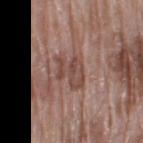Impression: Part of a total-body skin-imaging series; this lesion was reviewed on a skin check and was not flagged for biopsy. Acquisition and patient details: Longest diameter approximately 4 mm. The tile uses white-light illumination. A female patient, about 75 years old. From the right thigh. Automated tile analysis of the lesion measured a footprint of about 8 mm² and a shape-asymmetry score of about 0.4 (0 = symmetric). The analysis additionally found a lesion color around L≈46 a*≈20 b*≈24 in CIELAB, about 8 CIELAB-L* units darker than the surrounding skin, and a normalized border contrast of about 6.5. And it measured a border-irregularity rating of about 6/10 and a color-variation rating of about 3/10. Cropped from a whole-body photographic skin survey; the tile spans about 15 mm.